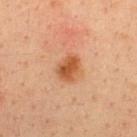Part of a total-body skin-imaging series; this lesion was reviewed on a skin check and was not flagged for biopsy. A male subject, aged approximately 35. Located on the upper back. Cropped from a total-body skin-imaging series; the visible field is about 15 mm.